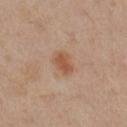biopsy status = catalogued during a skin exam; not biopsied
location = the left leg
image = ~15 mm crop, total-body skin-cancer survey
TBP lesion metrics = a nevus-likeness score of about 95/100 and a detector confidence of about 100 out of 100 that the crop contains a lesion
subject = female, aged approximately 40